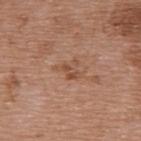biopsy status: no biopsy performed (imaged during a skin exam); subject: female, aged 68 to 72; TBP lesion metrics: an area of roughly 4 mm², a shape eccentricity near 0.6, and two-axis asymmetry of about 0.65; acquisition: total-body-photography crop, ~15 mm field of view; location: the upper back.